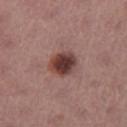image source = ~15 mm tile from a whole-body skin photo | patient = female, aged around 55 | location = the right thigh.